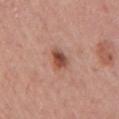Impression: Recorded during total-body skin imaging; not selected for excision or biopsy. Background: The lesion is located on the right upper arm. A region of skin cropped from a whole-body photographic capture, roughly 15 mm wide. A female subject aged 53 to 57.